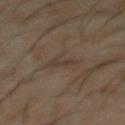Q: Was a biopsy performed?
A: catalogued during a skin exam; not biopsied
Q: What is the anatomic site?
A: the abdomen
Q: How was this image acquired?
A: ~15 mm tile from a whole-body skin photo
Q: What is the lesion's diameter?
A: about 3 mm
Q: What lighting was used for the tile?
A: cross-polarized illumination
Q: What did automated image analysis measure?
A: an area of roughly 3.5 mm², a shape eccentricity near 0.85, and a symmetry-axis asymmetry near 0.35; a lesion color around L≈34 a*≈11 b*≈21 in CIELAB, about 5 CIELAB-L* units darker than the surrounding skin, and a normalized border contrast of about 5; peripheral color asymmetry of about 0; a nevus-likeness score of about 0/100 and a detector confidence of about 70 out of 100 that the crop contains a lesion
Q: What are the patient's age and sex?
A: male, about 60 years old